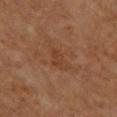The tile uses cross-polarized illumination. The lesion is on the upper back. Measured at roughly 4.5 mm in maximum diameter. A region of skin cropped from a whole-body photographic capture, roughly 15 mm wide. A male subject roughly 60 years of age.